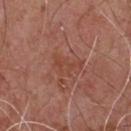- follow-up: imaged on a skin check; not biopsied
- anatomic site: the chest
- patient: male, aged around 55
- lighting: white-light
- acquisition: total-body-photography crop, ~15 mm field of view